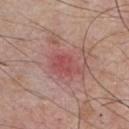<case>
  <biopsy_status>not biopsied; imaged during a skin examination</biopsy_status>
  <site>chest</site>
  <automated_metrics>
    <nevus_likeness_0_100>0</nevus_likeness_0_100>
    <lesion_detection_confidence_0_100>100</lesion_detection_confidence_0_100>
  </automated_metrics>
  <patient>
    <sex>male</sex>
    <age_approx>55</age_approx>
  </patient>
  <image>
    <source>total-body photography crop</source>
    <field_of_view_mm>15</field_of_view_mm>
  </image>
  <lighting>white-light</lighting>
</case>